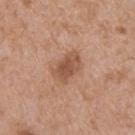Q: Was a biopsy performed?
A: imaged on a skin check; not biopsied
Q: What is the lesion's diameter?
A: ~4 mm (longest diameter)
Q: Illumination type?
A: white-light illumination
Q: What is the imaging modality?
A: 15 mm crop, total-body photography
Q: What are the patient's age and sex?
A: male, aged around 65
Q: What is the anatomic site?
A: the upper back